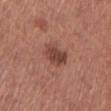Captured during whole-body skin photography for melanoma surveillance; the lesion was not biopsied. The patient is a female aged 63–67. From the leg. A region of skin cropped from a whole-body photographic capture, roughly 15 mm wide. Automated tile analysis of the lesion measured a mean CIELAB color near L≈44 a*≈23 b*≈26, about 10 CIELAB-L* units darker than the surrounding skin, and a lesion-to-skin contrast of about 8 (normalized; higher = more distinct). The analysis additionally found a classifier nevus-likeness of about 75/100 and a lesion-detection confidence of about 100/100.